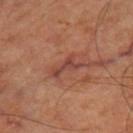  biopsy_status: not biopsied; imaged during a skin examination
  patient:
    age_approx: 65
  lighting: cross-polarized
  lesion_size:
    long_diameter_mm_approx: 4.0
  image:
    source: total-body photography crop
    field_of_view_mm: 15
  site: right thigh